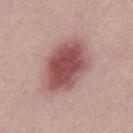imaging modality: 15 mm crop, total-body photography | patient: male, aged 28–32 | lighting: white-light | size: about 7.5 mm | TBP lesion metrics: an area of roughly 24 mm², an eccentricity of roughly 0.8, and a symmetry-axis asymmetry near 0.15; a mean CIELAB color near L≈51 a*≈24 b*≈21, about 15 CIELAB-L* units darker than the surrounding skin, and a normalized border contrast of about 10; a detector confidence of about 100 out of 100 that the crop contains a lesion.